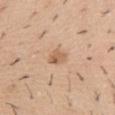Impression: Recorded during total-body skin imaging; not selected for excision or biopsy. Clinical summary: The lesion's longest dimension is about 2.5 mm. A male patient, aged 38 to 42. Captured under white-light illumination. This image is a 15 mm lesion crop taken from a total-body photograph. The lesion is on the abdomen.